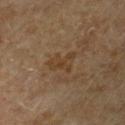The patient is a male aged around 65.
A 15 mm close-up extracted from a 3D total-body photography capture.
Located on the chest.
The total-body-photography lesion software estimated an area of roughly 6 mm², an eccentricity of roughly 0.7, and two-axis asymmetry of about 0.45. It also reported an average lesion color of about L≈34 a*≈14 b*≈28 (CIELAB), a lesion–skin lightness drop of about 5, and a normalized border contrast of about 6.
The tile uses cross-polarized illumination.